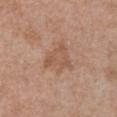workup: imaged on a skin check; not biopsied | lesion diameter: ≈4 mm | patient: male, in their 70s | site: the abdomen | image: total-body-photography crop, ~15 mm field of view.